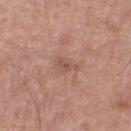The lesion was photographed on a routine skin check and not biopsied; there is no pathology result.
The recorded lesion diameter is about 2.5 mm.
This is a white-light tile.
A male patient, aged approximately 60.
Located on the right lower leg.
A region of skin cropped from a whole-body photographic capture, roughly 15 mm wide.